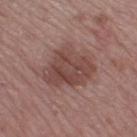biopsy_status: not biopsied; imaged during a skin examination
lesion_size:
  long_diameter_mm_approx: 6.0
automated_metrics:
  area_mm2_approx: 21.0
  eccentricity: 0.55
  shape_asymmetry: 0.25
site: right thigh
lighting: white-light
image:
  source: total-body photography crop
  field_of_view_mm: 15
patient:
  sex: male
  age_approx: 55